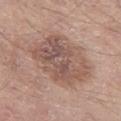Findings:
- acquisition: ~15 mm tile from a whole-body skin photo
- subject: male, in their mid- to late 60s
- anatomic site: the left thigh